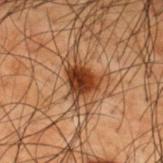Q: Where on the body is the lesion?
A: the upper back
Q: What is the imaging modality?
A: ~15 mm crop, total-body skin-cancer survey
Q: How large is the lesion?
A: about 3.5 mm
Q: Who is the patient?
A: male, roughly 50 years of age
Q: What did automated image analysis measure?
A: an eccentricity of roughly 0.55 and a shape-asymmetry score of about 0.35 (0 = symmetric); an automated nevus-likeness rating near 95 out of 100 and a detector confidence of about 100 out of 100 that the crop contains a lesion
Q: What lighting was used for the tile?
A: cross-polarized